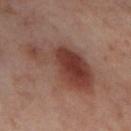Assessment: The lesion was photographed on a routine skin check and not biopsied; there is no pathology result. Acquisition and patient details: A female subject, aged 53 to 57. From the leg. Approximately 9 mm at its widest. A region of skin cropped from a whole-body photographic capture, roughly 15 mm wide.